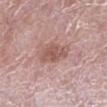{
  "biopsy_status": "not biopsied; imaged during a skin examination",
  "image": {
    "source": "total-body photography crop",
    "field_of_view_mm": 15
  },
  "patient": {
    "sex": "male",
    "age_approx": 50
  },
  "site": "leg"
}